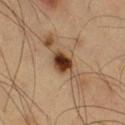The lesion was photographed on a routine skin check and not biopsied; there is no pathology result.
Automated image analysis of the tile measured a footprint of about 5.5 mm², a shape eccentricity near 0.5, and a shape-asymmetry score of about 0.25 (0 = symmetric). The software also gave a border-irregularity rating of about 2/10, internal color variation of about 5 on a 0–10 scale, and peripheral color asymmetry of about 1.5.
About 2.5 mm across.
This is a cross-polarized tile.
The patient is a male aged 58–62.
On the right thigh.
A roughly 15 mm field-of-view crop from a total-body skin photograph.